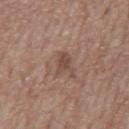Clinical impression:
No biopsy was performed on this lesion — it was imaged during a full skin examination and was not determined to be concerning.
Context:
Measured at roughly 3 mm in maximum diameter. A close-up tile cropped from a whole-body skin photograph, about 15 mm across. A male patient in their 70s. The lesion is on the mid back. This is a white-light tile.